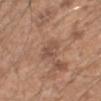Case summary:
• follow-up — total-body-photography surveillance lesion; no biopsy
• subject — male, aged approximately 50
• TBP lesion metrics — a lesion area of about 5.5 mm², an eccentricity of roughly 0.6, and a shape-asymmetry score of about 0.35 (0 = symmetric); a mean CIELAB color near L≈51 a*≈19 b*≈28, about 7 CIELAB-L* units darker than the surrounding skin, and a lesion-to-skin contrast of about 5.5 (normalized; higher = more distinct); a border-irregularity rating of about 3.5/10, a color-variation rating of about 2.5/10, and a peripheral color-asymmetry measure near 1
• illumination — white-light
• site — the left upper arm
• acquisition — total-body-photography crop, ~15 mm field of view
• diameter — about 3 mm